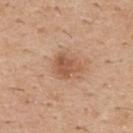This lesion was catalogued during total-body skin photography and was not selected for biopsy. Imaged with white-light lighting. On the upper back. Cropped from a total-body skin-imaging series; the visible field is about 15 mm. The patient is a male in their 40s. Approximately 3 mm at its widest. Automated image analysis of the tile measured a footprint of about 7 mm², an outline eccentricity of about 0.5 (0 = round, 1 = elongated), and a symmetry-axis asymmetry near 0.35. The software also gave an automated nevus-likeness rating near 45 out of 100 and a lesion-detection confidence of about 100/100.